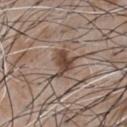Notes:
- follow-up — catalogued during a skin exam; not biopsied
- site — the chest
- patient — male, aged approximately 50
- tile lighting — white-light
- diameter — ~4 mm (longest diameter)
- image — ~15 mm crop, total-body skin-cancer survey